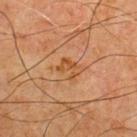{
  "biopsy_status": "not biopsied; imaged during a skin examination",
  "patient": {
    "sex": "male",
    "age_approx": 65
  },
  "image": {
    "source": "total-body photography crop",
    "field_of_view_mm": 15
  },
  "site": "chest"
}